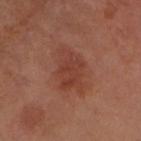A 15 mm close-up extracted from a 3D total-body photography capture. About 5.5 mm across. An algorithmic analysis of the crop reported a footprint of about 14 mm², a shape eccentricity near 0.75, and two-axis asymmetry of about 0.35. And it measured border irregularity of about 4 on a 0–10 scale and peripheral color asymmetry of about 1. A male subject, aged around 70. The tile uses cross-polarized illumination. The lesion is located on the arm.